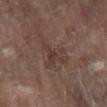| field | value |
|---|---|
| workup | catalogued during a skin exam; not biopsied |
| body site | the leg |
| TBP lesion metrics | about 6 CIELAB-L* units darker than the surrounding skin and a lesion-to-skin contrast of about 5.5 (normalized; higher = more distinct) |
| size | ~6.5 mm (longest diameter) |
| patient | male, aged around 70 |
| image | ~15 mm tile from a whole-body skin photo |